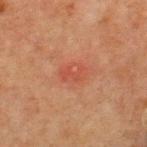Clinical impression: The lesion was photographed on a routine skin check and not biopsied; there is no pathology result. Context: A male patient, roughly 65 years of age. The lesion-visualizer software estimated a border-irregularity rating of about 2/10, a color-variation rating of about 2.5/10, and peripheral color asymmetry of about 1. The analysis additionally found a nevus-likeness score of about 0/100 and lesion-presence confidence of about 100/100. About 3 mm across. From the chest. Cropped from a whole-body photographic skin survey; the tile spans about 15 mm. Imaged with cross-polarized lighting.